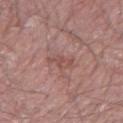Image and clinical context: The lesion is located on the right forearm. The patient is a male aged 63 to 67. Cropped from a whole-body photographic skin survey; the tile spans about 15 mm. Automated tile analysis of the lesion measured a mean CIELAB color near L≈50 a*≈22 b*≈23, roughly 7 lightness units darker than nearby skin, and a normalized lesion–skin contrast near 5.5. And it measured a border-irregularity index near 4/10 and a peripheral color-asymmetry measure near 0.5. The lesion's longest dimension is about 3.5 mm.